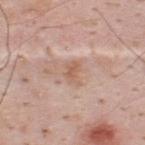Notes:
* follow-up: imaged on a skin check; not biopsied
* illumination: white-light illumination
* diameter: ~2.5 mm (longest diameter)
* image: total-body-photography crop, ~15 mm field of view
* patient: male, in their mid- to late 30s
* site: the upper back